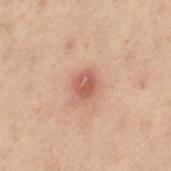A roughly 15 mm field-of-view crop from a total-body skin photograph. The recorded lesion diameter is about 2.5 mm. This is a cross-polarized tile. The lesion is on the left thigh. A female subject, aged around 55.